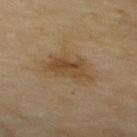<lesion>
  <biopsy_status>not biopsied; imaged during a skin examination</biopsy_status>
  <site>upper back</site>
  <patient>
    <sex>female</sex>
    <age_approx>60</age_approx>
  </patient>
  <image>
    <source>total-body photography crop</source>
    <field_of_view_mm>15</field_of_view_mm>
  </image>
</lesion>